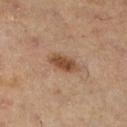workup: catalogued during a skin exam; not biopsied
patient: female, about 50 years old
lesion diameter: ~3.5 mm (longest diameter)
image source: ~15 mm crop, total-body skin-cancer survey
location: the right lower leg
lighting: cross-polarized
TBP lesion metrics: a footprint of about 5.5 mm², a shape eccentricity near 0.85, and two-axis asymmetry of about 0.3